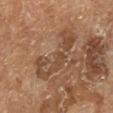Assessment: Recorded during total-body skin imaging; not selected for excision or biopsy. Clinical summary: Longest diameter approximately 7.5 mm. A male patient in their mid- to late 60s. A 15 mm close-up extracted from a 3D total-body photography capture. The lesion-visualizer software estimated a mean CIELAB color near L≈47 a*≈19 b*≈31, roughly 8 lightness units darker than nearby skin, and a normalized lesion–skin contrast near 6. The software also gave border irregularity of about 8.5 on a 0–10 scale, a within-lesion color-variation index near 5/10, and peripheral color asymmetry of about 1.5. The software also gave an automated nevus-likeness rating near 0 out of 100 and a lesion-detection confidence of about 95/100. Located on the right lower leg.